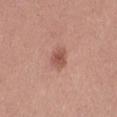biopsy status: imaged on a skin check; not biopsied | body site: the left thigh | subject: female, aged 23–27 | acquisition: ~15 mm crop, total-body skin-cancer survey.